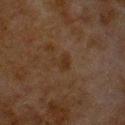Notes:
– follow-up · no biopsy performed (imaged during a skin exam)
– diameter · about 2.5 mm
– anatomic site · the chest
– lighting · cross-polarized
– imaging modality · total-body-photography crop, ~15 mm field of view
– subject · male, about 80 years old